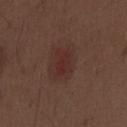Captured under white-light illumination. An algorithmic analysis of the crop reported a lesion area of about 5.5 mm², an outline eccentricity of about 0.75 (0 = round, 1 = elongated), and a symmetry-axis asymmetry near 0.25. The analysis additionally found an automated nevus-likeness rating near 60 out of 100 and lesion-presence confidence of about 100/100. A male subject, about 70 years old. A 15 mm close-up extracted from a 3D total-body photography capture. Located on the abdomen.